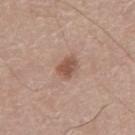<record>
  <biopsy_status>not biopsied; imaged during a skin examination</biopsy_status>
  <patient>
    <sex>male</sex>
    <age_approx>80</age_approx>
  </patient>
  <lesion_size>
    <long_diameter_mm_approx>3.0</long_diameter_mm_approx>
  </lesion_size>
  <image>
    <source>total-body photography crop</source>
    <field_of_view_mm>15</field_of_view_mm>
  </image>
  <automated_metrics>
    <area_mm2_approx>5.0</area_mm2_approx>
    <eccentricity>0.7</eccentricity>
    <shape_asymmetry>0.15</shape_asymmetry>
    <color_variation_0_10>3.5</color_variation_0_10>
    <peripheral_color_asymmetry>1.0</peripheral_color_asymmetry>
  </automated_metrics>
  <lighting>white-light</lighting>
  <site>abdomen</site>
</record>